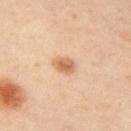Assessment: Part of a total-body skin-imaging series; this lesion was reviewed on a skin check and was not flagged for biopsy. Context: The patient is a female roughly 40 years of age. A roughly 15 mm field-of-view crop from a total-body skin photograph. An algorithmic analysis of the crop reported a lesion area of about 5 mm², a shape eccentricity near 0.7, and a shape-asymmetry score of about 0.15 (0 = symmetric). The software also gave a lesion color around L≈67 a*≈20 b*≈38 in CIELAB, about 12 CIELAB-L* units darker than the surrounding skin, and a lesion-to-skin contrast of about 7.5 (normalized; higher = more distinct). The analysis additionally found a classifier nevus-likeness of about 95/100 and a lesion-detection confidence of about 100/100. On the left arm. This is a cross-polarized tile.